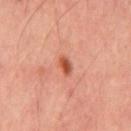Q: How was this image acquired?
A: 15 mm crop, total-body photography
Q: What are the patient's age and sex?
A: male, aged around 45
Q: How was the tile lit?
A: cross-polarized
Q: What is the anatomic site?
A: the back